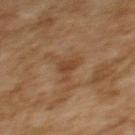| feature | finding |
|---|---|
| workup | total-body-photography surveillance lesion; no biopsy |
| location | the upper back |
| patient | female, roughly 60 years of age |
| acquisition | ~15 mm crop, total-body skin-cancer survey |